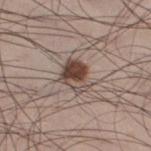Case summary:
– follow-up · catalogued during a skin exam; not biopsied
– patient · male, about 35 years old
– TBP lesion metrics · an area of roughly 9 mm², a shape eccentricity near 0.65, and a shape-asymmetry score of about 0.25 (0 = symmetric); a classifier nevus-likeness of about 100/100 and a detector confidence of about 100 out of 100 that the crop contains a lesion
– acquisition · ~15 mm tile from a whole-body skin photo
– diameter · ~3.5 mm (longest diameter)
– location · the right thigh